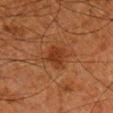Assessment: Imaged during a routine full-body skin examination; the lesion was not biopsied and no histopathology is available. Context: The total-body-photography lesion software estimated an eccentricity of roughly 0.7 and a shape-asymmetry score of about 0.3 (0 = symmetric). It also reported a classifier nevus-likeness of about 50/100 and a detector confidence of about 100 out of 100 that the crop contains a lesion. This is a cross-polarized tile. Longest diameter approximately 3 mm. The subject is a male roughly 80 years of age. A 15 mm crop from a total-body photograph taken for skin-cancer surveillance. The lesion is on the right lower leg.